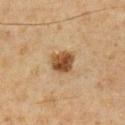{"biopsy_status": "not biopsied; imaged during a skin examination", "automated_metrics": {"border_irregularity_0_10": 1.5, "color_variation_0_10": 4.5, "nevus_likeness_0_100": 95, "lesion_detection_confidence_0_100": 100}, "lighting": "cross-polarized", "lesion_size": {"long_diameter_mm_approx": 3.0}, "image": {"source": "total-body photography crop", "field_of_view_mm": 15}, "site": "chest", "patient": {"sex": "male", "age_approx": 60}}